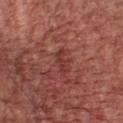Imaged during a routine full-body skin examination; the lesion was not biopsied and no histopathology is available. The tile uses white-light illumination. A roughly 15 mm field-of-view crop from a total-body skin photograph. A male patient aged around 65. The recorded lesion diameter is about 3 mm. The lesion is located on the chest.